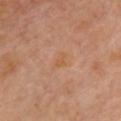Part of a total-body skin-imaging series; this lesion was reviewed on a skin check and was not flagged for biopsy. Longest diameter approximately 2.5 mm. Located on the chest. The tile uses cross-polarized illumination. The patient is a female approximately 60 years of age. A region of skin cropped from a whole-body photographic capture, roughly 15 mm wide.